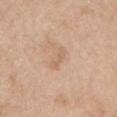Impression:
The lesion was photographed on a routine skin check and not biopsied; there is no pathology result.
Background:
Measured at roughly 3 mm in maximum diameter. A close-up tile cropped from a whole-body skin photograph, about 15 mm across. A male subject in their 60s. The lesion is located on the left upper arm.